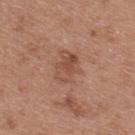Captured during whole-body skin photography for melanoma surveillance; the lesion was not biopsied.
From the upper back.
A female patient, roughly 40 years of age.
About 3.5 mm across.
A 15 mm close-up extracted from a 3D total-body photography capture.
The total-body-photography lesion software estimated border irregularity of about 3 on a 0–10 scale, internal color variation of about 5.5 on a 0–10 scale, and peripheral color asymmetry of about 2. The software also gave an automated nevus-likeness rating near 0 out of 100 and a lesion-detection confidence of about 100/100.
This is a white-light tile.